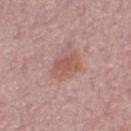Notes:
• biopsy status — total-body-photography surveillance lesion; no biopsy
• subject — female, aged approximately 50
• anatomic site — the left thigh
• tile lighting — white-light illumination
• imaging modality — total-body-photography crop, ~15 mm field of view
• diameter — about 3 mm
• image-analysis metrics — a shape eccentricity near 0.7 and a symmetry-axis asymmetry near 0.25; a lesion–skin lightness drop of about 8; lesion-presence confidence of about 100/100